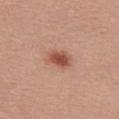Clinical impression: The lesion was photographed on a routine skin check and not biopsied; there is no pathology result. Background: Automated tile analysis of the lesion measured a lesion color around L≈52 a*≈25 b*≈30 in CIELAB, a lesion–skin lightness drop of about 12, and a normalized border contrast of about 8.5. The analysis additionally found a border-irregularity rating of about 2/10 and peripheral color asymmetry of about 1. The software also gave an automated nevus-likeness rating near 100 out of 100 and lesion-presence confidence of about 100/100. A region of skin cropped from a whole-body photographic capture, roughly 15 mm wide. Measured at roughly 3 mm in maximum diameter. The lesion is on the back. A female patient in their 20s.